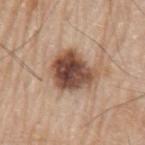Assessment: This lesion was catalogued during total-body skin photography and was not selected for biopsy. Acquisition and patient details: A 15 mm close-up tile from a total-body photography series done for melanoma screening. Captured under white-light illumination. A male subject, about 80 years old. The lesion is located on the arm.